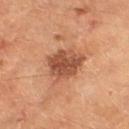Image and clinical context: This is a cross-polarized tile. The recorded lesion diameter is about 4.5 mm. From the left thigh. A male patient, aged 58 to 62. A 15 mm close-up tile from a total-body photography series done for melanoma screening.